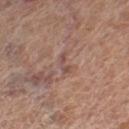notes: catalogued during a skin exam; not biopsied | illumination: white-light illumination | automated lesion analysis: a footprint of about 2 mm², an eccentricity of roughly 0.95, and a symmetry-axis asymmetry near 0.5; a lesion color around L≈49 a*≈20 b*≈23 in CIELAB and about 8 CIELAB-L* units darker than the surrounding skin; internal color variation of about 0 on a 0–10 scale and a peripheral color-asymmetry measure near 0 | image source: ~15 mm crop, total-body skin-cancer survey | site: the leg | patient: female, approximately 55 years of age | lesion diameter: ≈2.5 mm.